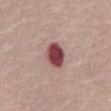{
  "biopsy_status": "not biopsied; imaged during a skin examination",
  "site": "abdomen",
  "lesion_size": {
    "long_diameter_mm_approx": 3.5
  },
  "patient": {
    "sex": "female",
    "age_approx": 65
  },
  "automated_metrics": {
    "area_mm2_approx": 7.5,
    "eccentricity": 0.55,
    "shape_asymmetry": 0.15,
    "cielab_L": 45,
    "cielab_a": 28,
    "cielab_b": 19,
    "vs_skin_darker_L": 19.0,
    "vs_skin_contrast_norm": 13.5,
    "border_irregularity_0_10": 1.5,
    "color_variation_0_10": 3.5,
    "peripheral_color_asymmetry": 1.0,
    "nevus_likeness_0_100": 10
  },
  "image": {
    "source": "total-body photography crop",
    "field_of_view_mm": 15
  }
}